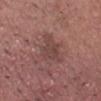  site: head or neck
  patient:
    sex: male
    age_approx: 25
  lesion_size:
    long_diameter_mm_approx: 4.5
  lighting: white-light
  automated_metrics:
    area_mm2_approx: 7.5
    shape_asymmetry: 0.45
    cielab_L: 43
    cielab_a: 20
    cielab_b: 21
    vs_skin_darker_L: 7.0
    border_irregularity_0_10: 5.5
    peripheral_color_asymmetry: 1.0
  image:
    source: total-body photography crop
    field_of_view_mm: 15